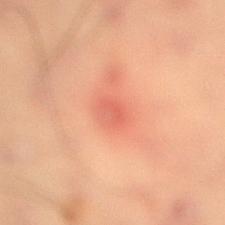| feature | finding |
|---|---|
| notes | imaged on a skin check; not biopsied |
| lighting | cross-polarized |
| subject | male, in their mid- to late 50s |
| image source | 15 mm crop, total-body photography |
| site | the right lower leg |